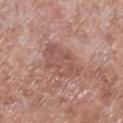{"biopsy_status": "not biopsied; imaged during a skin examination", "image": {"source": "total-body photography crop", "field_of_view_mm": 15}, "patient": {"sex": "male", "age_approx": 55}, "site": "right lower leg"}